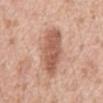This lesion was catalogued during total-body skin photography and was not selected for biopsy. This image is a 15 mm lesion crop taken from a total-body photograph. This is a white-light tile. The subject is a male about 60 years old. From the abdomen. The total-body-photography lesion software estimated a footprint of about 18 mm² and an eccentricity of roughly 0.9. The software also gave border irregularity of about 2.5 on a 0–10 scale and peripheral color asymmetry of about 1.5. And it measured a nevus-likeness score of about 65/100.